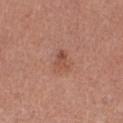Assessment: Part of a total-body skin-imaging series; this lesion was reviewed on a skin check and was not flagged for biopsy. Context: A female patient aged 58–62. The lesion is located on the right thigh. About 2.5 mm across. A 15 mm close-up extracted from a 3D total-body photography capture. Imaged with white-light lighting.